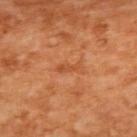Recorded during total-body skin imaging; not selected for excision or biopsy. A female patient in their mid-50s. The lesion is located on the upper back. The tile uses cross-polarized illumination. The recorded lesion diameter is about 2.5 mm. A lesion tile, about 15 mm wide, cut from a 3D total-body photograph.